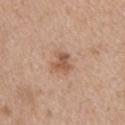<case>
  <biopsy_status>not biopsied; imaged during a skin examination</biopsy_status>
  <lesion_size>
    <long_diameter_mm_approx>2.5</long_diameter_mm_approx>
  </lesion_size>
  <lighting>white-light</lighting>
  <site>back</site>
  <image>
    <source>total-body photography crop</source>
    <field_of_view_mm>15</field_of_view_mm>
  </image>
  <patient>
    <sex>female</sex>
    <age_approx>45</age_approx>
  </patient>
</case>